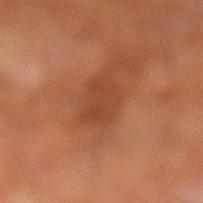workup: imaged on a skin check; not biopsied
automated lesion analysis: a footprint of about 7 mm², an eccentricity of roughly 0.85, and two-axis asymmetry of about 0.35; an average lesion color of about L≈34 a*≈22 b*≈29 (CIELAB), a lesion–skin lightness drop of about 6, and a lesion-to-skin contrast of about 6 (normalized; higher = more distinct); a border-irregularity rating of about 3.5/10, a within-lesion color-variation index near 1.5/10, and a peripheral color-asymmetry measure near 0.5
subject: male, aged 63 to 67
location: the left lower leg
illumination: cross-polarized
size: ~4 mm (longest diameter)
image: ~15 mm crop, total-body skin-cancer survey